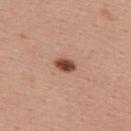Notes:
- biopsy status · no biopsy performed (imaged during a skin exam)
- anatomic site · the upper back
- imaging modality · total-body-photography crop, ~15 mm field of view
- patient · female, about 55 years old
- size · ≈2.5 mm